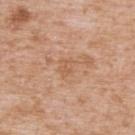Part of a total-body skin-imaging series; this lesion was reviewed on a skin check and was not flagged for biopsy. Located on the back. A region of skin cropped from a whole-body photographic capture, roughly 15 mm wide. A male patient in their mid-60s.